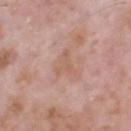Recorded during total-body skin imaging; not selected for excision or biopsy. The patient is a male about 70 years old. A 15 mm close-up tile from a total-body photography series done for melanoma screening. Automated tile analysis of the lesion measured roughly 6 lightness units darker than nearby skin and a lesion-to-skin contrast of about 4.5 (normalized; higher = more distinct). Longest diameter approximately 3.5 mm. From the head or neck. Imaged with white-light lighting.